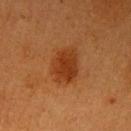Clinical impression:
Captured during whole-body skin photography for melanoma surveillance; the lesion was not biopsied.
Context:
The tile uses cross-polarized illumination. The recorded lesion diameter is about 4 mm. A female subject aged 53 to 57. The lesion-visualizer software estimated an average lesion color of about L≈32 a*≈25 b*≈34 (CIELAB). The software also gave a border-irregularity rating of about 2/10. The analysis additionally found a classifier nevus-likeness of about 100/100 and lesion-presence confidence of about 100/100. On the left upper arm. Cropped from a total-body skin-imaging series; the visible field is about 15 mm.